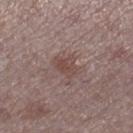Recorded during total-body skin imaging; not selected for excision or biopsy.
Longest diameter approximately 3 mm.
The tile uses white-light illumination.
Cropped from a whole-body photographic skin survey; the tile spans about 15 mm.
A female subject approximately 40 years of age.
On the right lower leg.